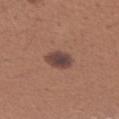Captured during whole-body skin photography for melanoma surveillance; the lesion was not biopsied.
The lesion is located on the arm.
A female subject aged approximately 40.
A lesion tile, about 15 mm wide, cut from a 3D total-body photograph.